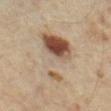No biopsy was performed on this lesion — it was imaged during a full skin examination and was not determined to be concerning.
The tile uses cross-polarized illumination.
A female patient, aged 33 to 37.
The lesion is on the leg.
A 15 mm close-up tile from a total-body photography series done for melanoma screening.
The recorded lesion diameter is about 8.5 mm.